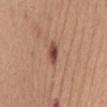biopsy_status: not biopsied; imaged during a skin examination
patient:
  sex: female
  age_approx: 50
image:
  source: total-body photography crop
  field_of_view_mm: 15
lesion_size:
  long_diameter_mm_approx: 3.5
site: mid back
automated_metrics:
  area_mm2_approx: 4.0
  eccentricity: 0.9
  shape_asymmetry: 0.2
  cielab_L: 47
  cielab_a: 22
  cielab_b: 28
  vs_skin_darker_L: 13.0
  vs_skin_contrast_norm: 9.5
  nevus_likeness_0_100: 95
  lesion_detection_confidence_0_100: 100